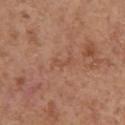Q: Was a biopsy performed?
A: no biopsy performed (imaged during a skin exam)
Q: What is the anatomic site?
A: the arm
Q: Who is the patient?
A: female, approximately 65 years of age
Q: What lighting was used for the tile?
A: white-light illumination
Q: Lesion size?
A: about 2.5 mm
Q: How was this image acquired?
A: ~15 mm crop, total-body skin-cancer survey